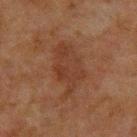The lesion was photographed on a routine skin check and not biopsied; there is no pathology result. The lesion is located on the upper back. A 15 mm close-up extracted from a 3D total-body photography capture. The subject is a male roughly 60 years of age.